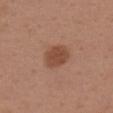{
  "biopsy_status": "not biopsied; imaged during a skin examination",
  "lighting": "white-light",
  "image": {
    "source": "total-body photography crop",
    "field_of_view_mm": 15
  },
  "patient": {
    "sex": "male",
    "age_approx": 40
  },
  "lesion_size": {
    "long_diameter_mm_approx": 3.0
  },
  "site": "upper back",
  "automated_metrics": {
    "area_mm2_approx": 7.5,
    "shape_asymmetry": 0.2,
    "cielab_L": 47,
    "cielab_a": 22,
    "cielab_b": 31,
    "vs_skin_darker_L": 10.0,
    "vs_skin_contrast_norm": 8.0,
    "nevus_likeness_0_100": 95,
    "lesion_detection_confidence_0_100": 100
  }
}